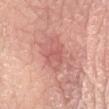The lesion was tiled from a total-body skin photograph and was not biopsied.
A female subject, aged 43–47.
On the left forearm.
A 15 mm close-up extracted from a 3D total-body photography capture.